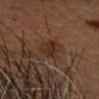- biopsy status: total-body-photography surveillance lesion; no biopsy